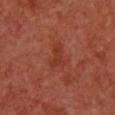Captured during whole-body skin photography for melanoma surveillance; the lesion was not biopsied. A roughly 15 mm field-of-view crop from a total-body skin photograph. The total-body-photography lesion software estimated an average lesion color of about L≈38 a*≈30 b*≈33 (CIELAB) and roughly 6 lightness units darker than nearby skin. The lesion is located on the chest. Imaged with cross-polarized lighting. Approximately 3 mm at its widest. A female patient aged 48 to 52.